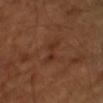Captured during whole-body skin photography for melanoma surveillance; the lesion was not biopsied. This image is a 15 mm lesion crop taken from a total-body photograph. A male subject, roughly 65 years of age.